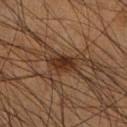{"biopsy_status": "not biopsied; imaged during a skin examination", "lighting": "cross-polarized", "patient": {"sex": "male", "age_approx": 45}, "image": {"source": "total-body photography crop", "field_of_view_mm": 15}, "site": "right lower leg", "automated_metrics": {"border_irregularity_0_10": 2.0, "color_variation_0_10": 3.5, "peripheral_color_asymmetry": 1.0}}